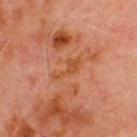Imaged during a routine full-body skin examination; the lesion was not biopsied and no histopathology is available.
The subject is a male aged around 70.
The lesion's longest dimension is about 4 mm.
The lesion is located on the chest.
A lesion tile, about 15 mm wide, cut from a 3D total-body photograph.
Imaged with cross-polarized lighting.